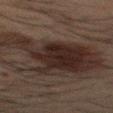Findings:
– follow-up · catalogued during a skin exam; not biopsied
– image · ~15 mm crop, total-body skin-cancer survey
– subject · male, roughly 50 years of age
– lesion size · ~11 mm (longest diameter)
– automated metrics · a footprint of about 44 mm², an eccentricity of roughly 0.8, and a shape-asymmetry score of about 0.55 (0 = symmetric); border irregularity of about 8 on a 0–10 scale, a within-lesion color-variation index near 4/10, and a peripheral color-asymmetry measure near 1
– body site · the mid back